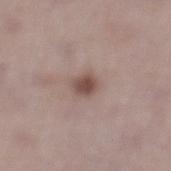biopsy status: no biopsy performed (imaged during a skin exam); imaging modality: 15 mm crop, total-body photography; location: the left lower leg; lesion size: ~2.5 mm (longest diameter); patient: female, aged around 50.